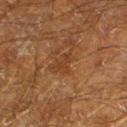Recorded during total-body skin imaging; not selected for excision or biopsy.
The recorded lesion diameter is about 3 mm.
Imaged with cross-polarized lighting.
A region of skin cropped from a whole-body photographic capture, roughly 15 mm wide.
A male patient, aged approximately 60.
On the left lower leg.